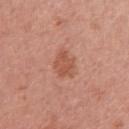Acquisition and patient details:
Imaged with white-light lighting. Approximately 3 mm at its widest. Located on the right upper arm. The lesion-visualizer software estimated an area of roughly 6.5 mm², an eccentricity of roughly 0.45, and a shape-asymmetry score of about 0.3 (0 = symmetric). The analysis additionally found a border-irregularity rating of about 3/10, a color-variation rating of about 2/10, and peripheral color asymmetry of about 0.5. And it measured a detector confidence of about 100 out of 100 that the crop contains a lesion. A female subject aged approximately 50. A 15 mm close-up extracted from a 3D total-body photography capture.